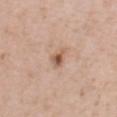About 2.5 mm across. The lesion-visualizer software estimated a lesion area of about 3 mm², an eccentricity of roughly 0.7, and two-axis asymmetry of about 0.35. And it measured a mean CIELAB color near L≈56 a*≈20 b*≈30 and a lesion-to-skin contrast of about 8 (normalized; higher = more distinct). The software also gave a classifier nevus-likeness of about 75/100 and lesion-presence confidence of about 100/100. Cropped from a total-body skin-imaging series; the visible field is about 15 mm. Imaged with white-light lighting. The patient is a female aged 38–42. Located on the chest.